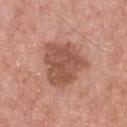biopsy status=total-body-photography surveillance lesion; no biopsy
patient=male, aged approximately 60
body site=the front of the torso
diameter=~5.5 mm (longest diameter)
automated lesion analysis=a border-irregularity index near 3/10, a color-variation rating of about 3/10, and peripheral color asymmetry of about 1; a detector confidence of about 100 out of 100 that the crop contains a lesion
image=~15 mm crop, total-body skin-cancer survey